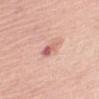Assessment: Captured during whole-body skin photography for melanoma surveillance; the lesion was not biopsied. Context: This is a white-light tile. A roughly 15 mm field-of-view crop from a total-body skin photograph. The lesion-visualizer software estimated an area of roughly 3.5 mm². The software also gave a mean CIELAB color near L≈62 a*≈27 b*≈25, roughly 12 lightness units darker than nearby skin, and a normalized border contrast of about 7.5. The lesion's longest dimension is about 3 mm. A female patient, about 65 years old. From the left upper arm.